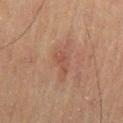Part of a total-body skin-imaging series; this lesion was reviewed on a skin check and was not flagged for biopsy. Cropped from a total-body skin-imaging series; the visible field is about 15 mm. The tile uses cross-polarized illumination. The patient is a male aged around 70. Located on the back. The lesion's longest dimension is about 3 mm.